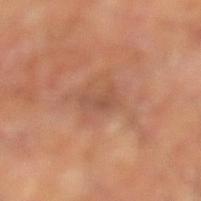workup=no biopsy performed (imaged during a skin exam)
lighting=cross-polarized
image-analysis metrics=an eccentricity of roughly 0.9 and a symmetry-axis asymmetry near 0.55; a lesion color around L≈49 a*≈22 b*≈30 in CIELAB and roughly 7 lightness units darker than nearby skin; a border-irregularity rating of about 6/10, a within-lesion color-variation index near 0/10, and a peripheral color-asymmetry measure near 0
patient=male, aged 68–72
body site=the leg
image=~15 mm tile from a whole-body skin photo
size=≈2.5 mm